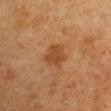| field | value |
|---|---|
| workup | no biopsy performed (imaged during a skin exam) |
| diameter | about 3.5 mm |
| patient | male, about 65 years old |
| tile lighting | cross-polarized |
| anatomic site | the right upper arm |
| image source | 15 mm crop, total-body photography |